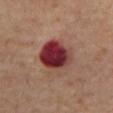Notes:
- biopsy status · catalogued during a skin exam; not biopsied
- tile lighting · cross-polarized
- subject · male, roughly 65 years of age
- lesion diameter · ~5 mm (longest diameter)
- imaging modality · 15 mm crop, total-body photography